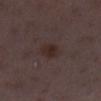{"biopsy_status": "not biopsied; imaged during a skin examination", "site": "left lower leg", "automated_metrics": {"cielab_L": 26, "cielab_a": 14, "cielab_b": 17, "vs_skin_darker_L": 6.0, "vs_skin_contrast_norm": 7.5, "nevus_likeness_0_100": 60}, "patient": {"sex": "female", "age_approx": 30}, "lighting": "white-light", "image": {"source": "total-body photography crop", "field_of_view_mm": 15}, "lesion_size": {"long_diameter_mm_approx": 2.5}}